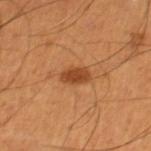biopsy_status: not biopsied; imaged during a skin examination
image:
  source: total-body photography crop
  field_of_view_mm: 15
site: leg
lighting: cross-polarized
patient:
  sex: male
  age_approx: 55
lesion_size:
  long_diameter_mm_approx: 3.0